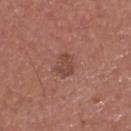The lesion was tiled from a total-body skin photograph and was not biopsied. The subject is a male aged around 45. The lesion is on the head or neck. Captured under white-light illumination. Approximately 3 mm at its widest. A 15 mm crop from a total-body photograph taken for skin-cancer surveillance.